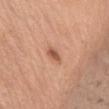The lesion was photographed on a routine skin check and not biopsied; there is no pathology result.